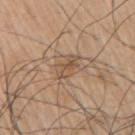<record>
  <biopsy_status>not biopsied; imaged during a skin examination</biopsy_status>
  <lesion_size>
    <long_diameter_mm_approx>3.5</long_diameter_mm_approx>
  </lesion_size>
  <image>
    <source>total-body photography crop</source>
    <field_of_view_mm>15</field_of_view_mm>
  </image>
  <patient>
    <sex>male</sex>
    <age_approx>75</age_approx>
  </patient>
  <site>arm</site>
  <lighting>white-light</lighting>
</record>